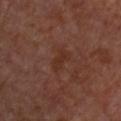| feature | finding |
|---|---|
| biopsy status | catalogued during a skin exam; not biopsied |
| subject | female, aged 58 to 62 |
| image | 15 mm crop, total-body photography |
| location | the front of the torso |
| automated metrics | a mean CIELAB color near L≈31 a*≈22 b*≈27, a lesion–skin lightness drop of about 5, and a normalized lesion–skin contrast near 6.5; a border-irregularity rating of about 3.5/10, internal color variation of about 2 on a 0–10 scale, and a peripheral color-asymmetry measure near 0.5 |